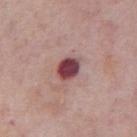Notes:
* notes · imaged on a skin check; not biopsied
* size · ≈3 mm
* subject · male, aged 73 to 77
* body site · the abdomen
* automated lesion analysis · a lesion–skin lightness drop of about 18; a classifier nevus-likeness of about 15/100
* tile lighting · white-light illumination
* imaging modality · 15 mm crop, total-body photography